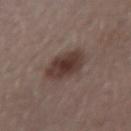biopsy status = imaged on a skin check; not biopsied
acquisition = ~15 mm tile from a whole-body skin photo
site = the right forearm
subject = female, aged approximately 55